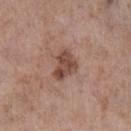Notes:
– notes · catalogued during a skin exam; not biopsied
– patient · female, in their mid-80s or older
– automated lesion analysis · border irregularity of about 3.5 on a 0–10 scale, internal color variation of about 4 on a 0–10 scale, and radial color variation of about 1.5
– location · the left lower leg
– imaging modality · ~15 mm crop, total-body skin-cancer survey
– diameter · ≈3.5 mm